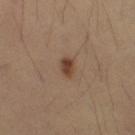Assessment:
The lesion was tiled from a total-body skin photograph and was not biopsied.
Acquisition and patient details:
The lesion's longest dimension is about 2.5 mm. The lesion is on the right thigh. A 15 mm crop from a total-body photograph taken for skin-cancer surveillance. The patient is a female aged 33–37. This is a cross-polarized tile.